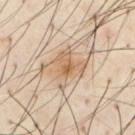body site: the chest
lighting: cross-polarized
patient: male, aged 53–57
lesion diameter: ~4.5 mm (longest diameter)
imaging modality: 15 mm crop, total-body photography